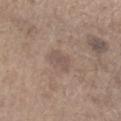{
  "biopsy_status": "not biopsied; imaged during a skin examination",
  "lesion_size": {
    "long_diameter_mm_approx": 3.0
  },
  "patient": {
    "sex": "male",
    "age_approx": 70
  },
  "image": {
    "source": "total-body photography crop",
    "field_of_view_mm": 15
  },
  "site": "arm"
}